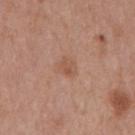Recorded during total-body skin imaging; not selected for excision or biopsy. About 2.5 mm across. The patient is a male aged approximately 70. A close-up tile cropped from a whole-body skin photograph, about 15 mm across. This is a white-light tile. An algorithmic analysis of the crop reported an average lesion color of about L≈54 a*≈21 b*≈30 (CIELAB) and a lesion–skin lightness drop of about 7. The software also gave a border-irregularity rating of about 2/10, a color-variation rating of about 2/10, and radial color variation of about 0.5. The analysis additionally found lesion-presence confidence of about 100/100. Located on the back.